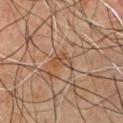biopsy status: total-body-photography surveillance lesion; no biopsy
location: the chest
patient: male, about 65 years old
imaging modality: ~15 mm crop, total-body skin-cancer survey
lesion size: about 2.5 mm
tile lighting: cross-polarized